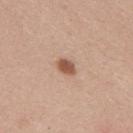biopsy_status: not biopsied; imaged during a skin examination
lighting: white-light
automated_metrics:
  cielab_L: 55
  cielab_a: 21
  cielab_b: 30
  vs_skin_darker_L: 13.0
  vs_skin_contrast_norm: 9.0
  border_irregularity_0_10: 1.5
  color_variation_0_10: 2.0
  peripheral_color_asymmetry: 0.5
patient:
  sex: male
  age_approx: 25
site: upper back
image:
  source: total-body photography crop
  field_of_view_mm: 15